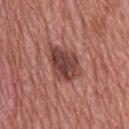notes — total-body-photography surveillance lesion; no biopsy | illumination — white-light | patient — male, aged 73 to 77 | automated metrics — a footprint of about 12 mm², an outline eccentricity of about 0.65 (0 = round, 1 = elongated), and a symmetry-axis asymmetry near 0.25; a border-irregularity index near 2.5/10, a within-lesion color-variation index near 4.5/10, and radial color variation of about 1.5; a classifier nevus-likeness of about 10/100 and lesion-presence confidence of about 100/100 | diameter — ≈4.5 mm | anatomic site — the upper back | image source — total-body-photography crop, ~15 mm field of view.